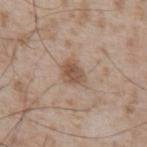Captured during whole-body skin photography for melanoma surveillance; the lesion was not biopsied.
Cropped from a total-body skin-imaging series; the visible field is about 15 mm.
A male patient, aged around 55.
The lesion is on the abdomen.